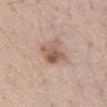{"biopsy_status": "not biopsied; imaged during a skin examination", "site": "lower back", "lesion_size": {"long_diameter_mm_approx": 3.5}, "image": {"source": "total-body photography crop", "field_of_view_mm": 15}, "lighting": "white-light", "patient": {"sex": "male", "age_approx": 30}}